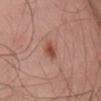| feature | finding |
|---|---|
| follow-up | imaged on a skin check; not biopsied |
| body site | the lower back |
| tile lighting | white-light illumination |
| subject | male, aged 53–57 |
| image source | total-body-photography crop, ~15 mm field of view |
| lesion diameter | about 3 mm |
| automated metrics | a lesion area of about 4.5 mm², a shape eccentricity near 0.85, and two-axis asymmetry of about 0.2; a border-irregularity rating of about 2/10, a color-variation rating of about 4/10, and peripheral color asymmetry of about 1.5; a lesion-detection confidence of about 100/100 |